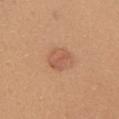Clinical impression:
No biopsy was performed on this lesion — it was imaged during a full skin examination and was not determined to be concerning.
Clinical summary:
The lesion's longest dimension is about 3 mm. Located on the front of the torso. A 15 mm crop from a total-body photograph taken for skin-cancer surveillance. The lesion-visualizer software estimated a lesion area of about 6.5 mm², an outline eccentricity of about 0.55 (0 = round, 1 = elongated), and a symmetry-axis asymmetry near 0.15. The software also gave about 8 CIELAB-L* units darker than the surrounding skin and a normalized border contrast of about 5.5. Captured under white-light illumination. A female subject, roughly 40 years of age.